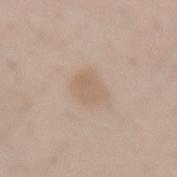Findings:
– biopsy status · total-body-photography surveillance lesion; no biopsy
– body site · the lower back
– illumination · white-light
– size · ~3.5 mm (longest diameter)
– image-analysis metrics · an area of roughly 7.5 mm²; an average lesion color of about L≈62 a*≈14 b*≈29 (CIELAB), roughly 6 lightness units darker than nearby skin, and a lesion-to-skin contrast of about 5 (normalized; higher = more distinct)
– patient · male, aged around 50
– imaging modality · total-body-photography crop, ~15 mm field of view